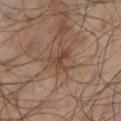Assessment:
This lesion was catalogued during total-body skin photography and was not selected for biopsy.
Clinical summary:
The tile uses cross-polarized illumination. A male patient, about 65 years old. The lesion is on the left lower leg. A 15 mm close-up tile from a total-body photography series done for melanoma screening.